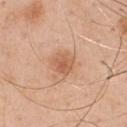Assessment:
This lesion was catalogued during total-body skin photography and was not selected for biopsy.
Acquisition and patient details:
A 15 mm close-up tile from a total-body photography series done for melanoma screening. Longest diameter approximately 3 mm. A male subject, roughly 50 years of age. Imaged with white-light lighting. The total-body-photography lesion software estimated border irregularity of about 1.5 on a 0–10 scale and a color-variation rating of about 3.5/10. The software also gave an automated nevus-likeness rating near 70 out of 100 and lesion-presence confidence of about 100/100. The lesion is located on the chest.